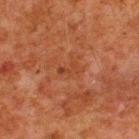Impression: The lesion was photographed on a routine skin check and not biopsied; there is no pathology result. Clinical summary: The patient is a male aged 78–82. Cropped from a whole-body photographic skin survey; the tile spans about 15 mm. On the upper back. Automated image analysis of the tile measured a symmetry-axis asymmetry near 0.45. The analysis additionally found a mean CIELAB color near L≈33 a*≈23 b*≈30, roughly 5 lightness units darker than nearby skin, and a normalized lesion–skin contrast near 5. It also reported a nevus-likeness score of about 0/100 and a lesion-detection confidence of about 100/100. The lesion's longest dimension is about 3 mm.